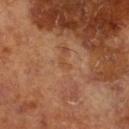The lesion-visualizer software estimated an area of roughly 1 mm², an outline eccentricity of about 0.9 (0 = round, 1 = elongated), and two-axis asymmetry of about 0.6. And it measured a border-irregularity index near 5.5/10, internal color variation of about 0 on a 0–10 scale, and peripheral color asymmetry of about 0. And it measured lesion-presence confidence of about 100/100.
A 15 mm close-up extracted from a 3D total-body photography capture.
The lesion's longest dimension is about 1.5 mm.
The lesion is on the right lower leg.
A male subject, roughly 65 years of age.